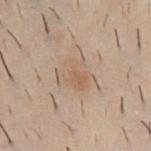{
  "biopsy_status": "not biopsied; imaged during a skin examination",
  "lighting": "cross-polarized",
  "lesion_size": {
    "long_diameter_mm_approx": 4.0
  },
  "image": {
    "source": "total-body photography crop",
    "field_of_view_mm": 15
  },
  "patient": {
    "sex": "male",
    "age_approx": 30
  },
  "automated_metrics": {
    "eccentricity": 0.65,
    "nevus_likeness_0_100": 10
  },
  "site": "chest"
}